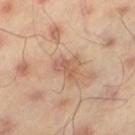biopsy status: imaged on a skin check; not biopsied
diameter: ≈3 mm
subject: male, aged 43–47
tile lighting: cross-polarized
image: ~15 mm crop, total-body skin-cancer survey
anatomic site: the leg